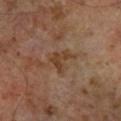This lesion was catalogued during total-body skin photography and was not selected for biopsy.
A male patient aged around 60.
Cropped from a total-body skin-imaging series; the visible field is about 15 mm.
Imaged with cross-polarized lighting.
The lesion is located on the right lower leg.
The recorded lesion diameter is about 3.5 mm.